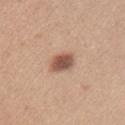follow-up=imaged on a skin check; not biopsied | subject=female, aged 23–27 | lesion size=~3 mm (longest diameter) | image=~15 mm crop, total-body skin-cancer survey | location=the right upper arm | lighting=white-light.